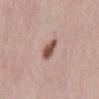Assessment:
Recorded during total-body skin imaging; not selected for excision or biopsy.
Image and clinical context:
Captured under white-light illumination. A female subject in their 30s. The recorded lesion diameter is about 3 mm. Cropped from a total-body skin-imaging series; the visible field is about 15 mm. The lesion is located on the front of the torso.